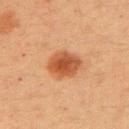Assessment:
The lesion was photographed on a routine skin check and not biopsied; there is no pathology result.
Clinical summary:
From the left upper arm. Automated image analysis of the tile measured border irregularity of about 2 on a 0–10 scale, a color-variation rating of about 4.5/10, and radial color variation of about 1. Captured under cross-polarized illumination. A lesion tile, about 15 mm wide, cut from a 3D total-body photograph. A female patient, roughly 40 years of age. The recorded lesion diameter is about 4.5 mm.